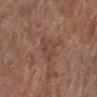Q: Was a biopsy performed?
A: total-body-photography surveillance lesion; no biopsy
Q: What kind of image is this?
A: 15 mm crop, total-body photography
Q: Patient demographics?
A: female, about 80 years old
Q: What did automated image analysis measure?
A: a lesion area of about 6.5 mm² and a shape eccentricity near 0.65
Q: Where on the body is the lesion?
A: the left lower leg
Q: Illumination type?
A: white-light illumination
Q: How large is the lesion?
A: ~3.5 mm (longest diameter)